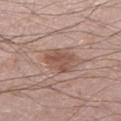| feature | finding |
|---|---|
| notes | no biopsy performed (imaged during a skin exam) |